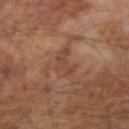Captured during whole-body skin photography for melanoma surveillance; the lesion was not biopsied. Approximately 4 mm at its widest. Automated image analysis of the tile measured a lesion area of about 5.5 mm², a shape eccentricity near 0.9, and a symmetry-axis asymmetry near 0.4. It also reported a border-irregularity index near 5/10, a color-variation rating of about 2/10, and radial color variation of about 0.5. The software also gave a classifier nevus-likeness of about 0/100 and a detector confidence of about 85 out of 100 that the crop contains a lesion. Located on the left arm. A 15 mm close-up extracted from a 3D total-body photography capture. This is a cross-polarized tile. A male patient aged approximately 65.